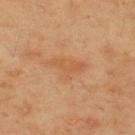{"biopsy_status": "not biopsied; imaged during a skin examination", "site": "upper back", "patient": {"sex": "male", "age_approx": 45}, "lesion_size": {"long_diameter_mm_approx": 5.0}, "image": {"source": "total-body photography crop", "field_of_view_mm": 15}, "lighting": "cross-polarized"}